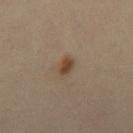Recorded during total-body skin imaging; not selected for excision or biopsy. The lesion is on the left leg. The tile uses cross-polarized illumination. A female patient roughly 40 years of age. A 15 mm close-up tile from a total-body photography series done for melanoma screening. The recorded lesion diameter is about 2.5 mm.